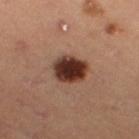Acquisition and patient details: A roughly 15 mm field-of-view crop from a total-body skin photograph. The lesion is on the right thigh. A female subject, roughly 45 years of age.